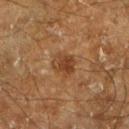Part of a total-body skin-imaging series; this lesion was reviewed on a skin check and was not flagged for biopsy. Measured at roughly 3 mm in maximum diameter. Located on the leg. A male patient, in their 60s. A lesion tile, about 15 mm wide, cut from a 3D total-body photograph. The tile uses cross-polarized illumination. The lesion-visualizer software estimated an average lesion color of about L≈31 a*≈17 b*≈27 (CIELAB) and a normalized border contrast of about 7.5. And it measured lesion-presence confidence of about 100/100.